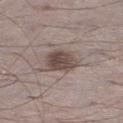{
  "biopsy_status": "not biopsied; imaged during a skin examination",
  "image": {
    "source": "total-body photography crop",
    "field_of_view_mm": 15
  },
  "site": "leg",
  "patient": {
    "sex": "male",
    "age_approx": 70
  },
  "lighting": "white-light"
}